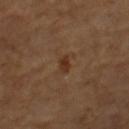Q: Is there a histopathology result?
A: imaged on a skin check; not biopsied
Q: What kind of image is this?
A: ~15 mm crop, total-body skin-cancer survey
Q: Who is the patient?
A: female, approximately 55 years of age
Q: What is the anatomic site?
A: the left thigh